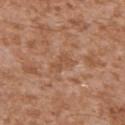This lesion was catalogued during total-body skin photography and was not selected for biopsy.
A male patient approximately 45 years of age.
Located on the left upper arm.
A roughly 15 mm field-of-view crop from a total-body skin photograph.